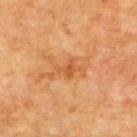– workup · total-body-photography surveillance lesion; no biopsy
– automated metrics · internal color variation of about 1 on a 0–10 scale; a nevus-likeness score of about 0/100
– anatomic site · the back
– image source · total-body-photography crop, ~15 mm field of view
– illumination · cross-polarized
– subject · male, aged around 60